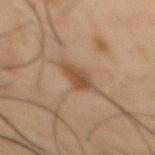Imaged during a routine full-body skin examination; the lesion was not biopsied and no histopathology is available. Located on the abdomen. A male subject roughly 50 years of age. This image is a 15 mm lesion crop taken from a total-body photograph. Automated image analysis of the tile measured a lesion area of about 5 mm², an eccentricity of roughly 0.55, and two-axis asymmetry of about 0.35. It also reported an automated nevus-likeness rating near 70 out of 100 and lesion-presence confidence of about 100/100. Captured under cross-polarized illumination.